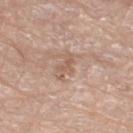<case>
<biopsy_status>not biopsied; imaged during a skin examination</biopsy_status>
<site>right thigh</site>
<patient>
  <sex>male</sex>
  <age_approx>80</age_approx>
</patient>
<lighting>white-light</lighting>
<lesion_size>
  <long_diameter_mm_approx>3.5</long_diameter_mm_approx>
</lesion_size>
<image>
  <source>total-body photography crop</source>
  <field_of_view_mm>15</field_of_view_mm>
</image>
</case>